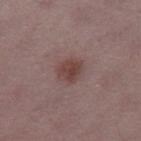{
  "biopsy_status": "not biopsied; imaged during a skin examination",
  "patient": {
    "sex": "female",
    "age_approx": 50
  },
  "image": {
    "source": "total-body photography crop",
    "field_of_view_mm": 15
  },
  "site": "right thigh"
}